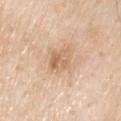The lesion was tiled from a total-body skin photograph and was not biopsied. A lesion tile, about 15 mm wide, cut from a 3D total-body photograph. From the chest. A male patient, about 80 years old. The tile uses white-light illumination. Automated tile analysis of the lesion measured a footprint of about 6 mm², a shape eccentricity near 0.75, and two-axis asymmetry of about 0.15. The software also gave a lesion color around L≈65 a*≈19 b*≈35 in CIELAB, roughly 10 lightness units darker than nearby skin, and a lesion-to-skin contrast of about 6.5 (normalized; higher = more distinct). And it measured a nevus-likeness score of about 5/100 and a detector confidence of about 100 out of 100 that the crop contains a lesion.